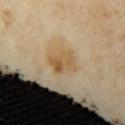Assessment: Recorded during total-body skin imaging; not selected for excision or biopsy. Background: A 15 mm crop from a total-body photograph taken for skin-cancer surveillance. A female subject, roughly 35 years of age. This is a cross-polarized tile. The lesion's longest dimension is about 3 mm. Automated tile analysis of the lesion measured a lesion area of about 7 mm² and a symmetry-axis asymmetry near 0.2. The software also gave an average lesion color of about L≈53 a*≈14 b*≈36 (CIELAB) and about 7 CIELAB-L* units darker than the surrounding skin. The analysis additionally found a border-irregularity index near 2.5/10, a color-variation rating of about 5.5/10, and radial color variation of about 2. The software also gave an automated nevus-likeness rating near 0 out of 100 and a detector confidence of about 100 out of 100 that the crop contains a lesion. Located on the left upper arm.